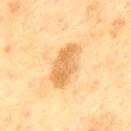A region of skin cropped from a whole-body photographic capture, roughly 15 mm wide.
A male patient aged approximately 70.
Located on the mid back.
This is a cross-polarized tile.